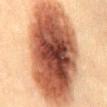Background:
The subject is a male aged 58 to 62. A lesion tile, about 15 mm wide, cut from a 3D total-body photograph. From the mid back.
Diagnosis:
On excision, pathology confirmed an invasive melanoma, superficial spreading type (Breslow thickness 0.3 mm, mitotic rate 0/mm²).